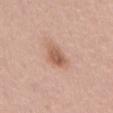Q: Was a biopsy performed?
A: imaged on a skin check; not biopsied
Q: What is the lesion's diameter?
A: about 4 mm
Q: Patient demographics?
A: male, roughly 55 years of age
Q: Lesion location?
A: the abdomen
Q: What kind of image is this?
A: total-body-photography crop, ~15 mm field of view